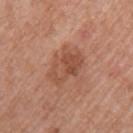lighting: white-light
body site: the left upper arm
subject: female, in their 60s
automated metrics: a nevus-likeness score of about 15/100 and a lesion-detection confidence of about 100/100
diameter: about 4.5 mm
acquisition: total-body-photography crop, ~15 mm field of view